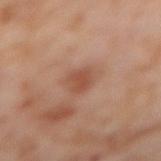workup: no biopsy performed (imaged during a skin exam)
patient: female, aged 53–57
tile lighting: cross-polarized
imaging modality: total-body-photography crop, ~15 mm field of view
location: the right thigh